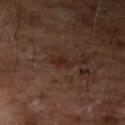Impression: This lesion was catalogued during total-body skin photography and was not selected for biopsy. Clinical summary: The lesion is located on the left forearm. The subject is a male aged 63–67. Longest diameter approximately 2.5 mm. The tile uses cross-polarized illumination. A region of skin cropped from a whole-body photographic capture, roughly 15 mm wide. The total-body-photography lesion software estimated two-axis asymmetry of about 0.3. It also reported an average lesion color of about L≈23 a*≈18 b*≈21 (CIELAB), a lesion–skin lightness drop of about 6, and a normalized lesion–skin contrast near 7.